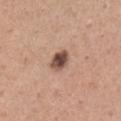| field | value |
|---|---|
| follow-up | total-body-photography surveillance lesion; no biopsy |
| image source | total-body-photography crop, ~15 mm field of view |
| location | the right upper arm |
| image-analysis metrics | a nevus-likeness score of about 80/100 and a detector confidence of about 100 out of 100 that the crop contains a lesion |
| tile lighting | white-light |
| lesion diameter | ≈3 mm |
| subject | male, about 45 years old |